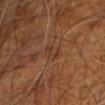biopsy status: no biopsy performed (imaged during a skin exam) | anatomic site: the arm | tile lighting: cross-polarized illumination | subject: male, aged 58 to 62 | image source: ~15 mm crop, total-body skin-cancer survey | lesion size: ≈2.5 mm.